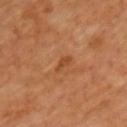The recorded lesion diameter is about 2.5 mm.
A male subject, aged 63–67.
Captured under cross-polarized illumination.
Cropped from a total-body skin-imaging series; the visible field is about 15 mm.
Located on the chest.